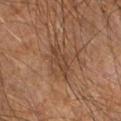workup = imaged on a skin check; not biopsied | image-analysis metrics = a lesion color around L≈41 a*≈18 b*≈30 in CIELAB and a lesion–skin lightness drop of about 7; a within-lesion color-variation index near 2.5/10 and a peripheral color-asymmetry measure near 1; a classifier nevus-likeness of about 0/100 and lesion-presence confidence of about 100/100 | image source = ~15 mm crop, total-body skin-cancer survey | subject = male, in their 60s | anatomic site = the right forearm | lesion size = about 4 mm.